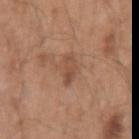follow-up: no biopsy performed (imaged during a skin exam)
lighting: white-light
location: the left upper arm
lesion diameter: about 2.5 mm
patient: male, in their 50s
image: ~15 mm crop, total-body skin-cancer survey